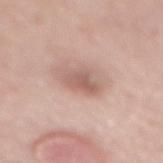{
  "biopsy_status": "not biopsied; imaged during a skin examination",
  "patient": {
    "sex": "female",
    "age_approx": 65
  },
  "image": {
    "source": "total-body photography crop",
    "field_of_view_mm": 15
  },
  "site": "front of the torso"
}